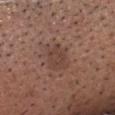Imaged with white-light lighting.
Longest diameter approximately 3.5 mm.
The lesion is on the head or neck.
A 15 mm close-up extracted from a 3D total-body photography capture.
The patient is a male roughly 65 years of age.
The lesion-visualizer software estimated a border-irregularity rating of about 3.5/10, a color-variation rating of about 2/10, and peripheral color asymmetry of about 0.5. It also reported a nevus-likeness score of about 0/100 and a detector confidence of about 100 out of 100 that the crop contains a lesion.